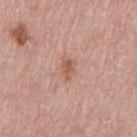Assessment:
Captured during whole-body skin photography for melanoma surveillance; the lesion was not biopsied.
Clinical summary:
The tile uses white-light illumination. A female subject in their mid-60s. The lesion-visualizer software estimated a footprint of about 3 mm². About 2.5 mm across. On the leg. A roughly 15 mm field-of-view crop from a total-body skin photograph.